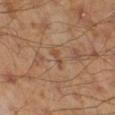{
  "biopsy_status": "not biopsied; imaged during a skin examination",
  "site": "right lower leg",
  "patient": {
    "sex": "male",
    "age_approx": 45
  },
  "image": {
    "source": "total-body photography crop",
    "field_of_view_mm": 15
  }
}